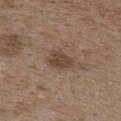Image and clinical context: The lesion is on the upper back. Imaged with white-light lighting. Automated tile analysis of the lesion measured an average lesion color of about L≈43 a*≈15 b*≈25 (CIELAB), about 10 CIELAB-L* units darker than the surrounding skin, and a normalized border contrast of about 8. It also reported a classifier nevus-likeness of about 75/100 and lesion-presence confidence of about 100/100. The lesion's longest dimension is about 3.5 mm. A female subject aged approximately 35. Cropped from a total-body skin-imaging series; the visible field is about 15 mm.